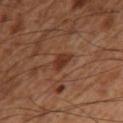workup = no biopsy performed (imaged during a skin exam)
image = ~15 mm tile from a whole-body skin photo
location = the right thigh
size = about 2.5 mm
patient = male, about 55 years old
illumination = cross-polarized